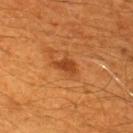Background: A male patient aged around 60. Cropped from a total-body skin-imaging series; the visible field is about 15 mm. From the upper back.